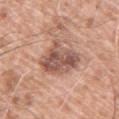biopsy_status: not biopsied; imaged during a skin examination
image:
  source: total-body photography crop
  field_of_view_mm: 15
patient:
  sex: male
  age_approx: 60
site: right upper arm
lesion_size:
  long_diameter_mm_approx: 5.5
lighting: white-light